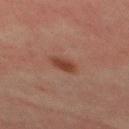biopsy_status: not biopsied; imaged during a skin examination
patient:
  sex: male
  age_approx: 30
image:
  source: total-body photography crop
  field_of_view_mm: 15
lighting: cross-polarized
automated_metrics:
  cielab_L: 33
  cielab_a: 20
  cielab_b: 25
  vs_skin_darker_L: 8.0
  vs_skin_contrast_norm: 8.5
site: mid back
lesion_size:
  long_diameter_mm_approx: 2.5